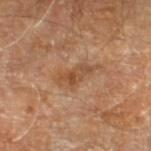Impression: Part of a total-body skin-imaging series; this lesion was reviewed on a skin check and was not flagged for biopsy. Context: From the left lower leg. A male patient aged 68 to 72. The total-body-photography lesion software estimated internal color variation of about 4.5 on a 0–10 scale and radial color variation of about 1.5. Longest diameter approximately 4.5 mm. Cropped from a whole-body photographic skin survey; the tile spans about 15 mm. The tile uses cross-polarized illumination.